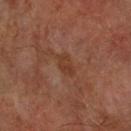biopsy status — imaged on a skin check; not biopsied | diameter — ≈2.5 mm | illumination — cross-polarized | image-analysis metrics — a classifier nevus-likeness of about 0/100 and lesion-presence confidence of about 100/100 | acquisition — ~15 mm crop, total-body skin-cancer survey | anatomic site — the right forearm | patient — male, in their mid-70s.